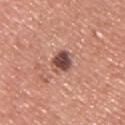Case summary:
* biopsy status — no biopsy performed (imaged during a skin exam)
* lesion size — ~3 mm (longest diameter)
* patient — male, aged 43–47
* body site — the upper back
* image source — 15 mm crop, total-body photography
* lighting — white-light